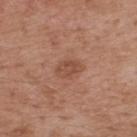| field | value |
|---|---|
| follow-up | catalogued during a skin exam; not biopsied |
| anatomic site | the upper back |
| lesion size | ~3 mm (longest diameter) |
| subject | male, in their mid-50s |
| automated metrics | a mean CIELAB color near L≈49 a*≈23 b*≈30 and about 8 CIELAB-L* units darker than the surrounding skin; a border-irregularity rating of about 2/10, a within-lesion color-variation index near 2/10, and peripheral color asymmetry of about 1 |
| imaging modality | ~15 mm tile from a whole-body skin photo |